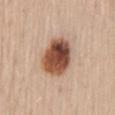The lesion was tiled from a total-body skin photograph and was not biopsied. Located on the mid back. Automated tile analysis of the lesion measured a lesion–skin lightness drop of about 20. And it measured a border-irregularity index near 2/10, a within-lesion color-variation index near 9.5/10, and a peripheral color-asymmetry measure near 3. Measured at roughly 5 mm in maximum diameter. Cropped from a whole-body photographic skin survey; the tile spans about 15 mm. Imaged with white-light lighting. A female patient, roughly 70 years of age.